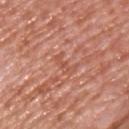No biopsy was performed on this lesion — it was imaged during a full skin examination and was not determined to be concerning. The subject is a male in their mid- to late 70s. The recorded lesion diameter is about 3 mm. Cropped from a whole-body photographic skin survey; the tile spans about 15 mm. Automated tile analysis of the lesion measured a footprint of about 3.5 mm² and an eccentricity of roughly 0.85. The software also gave roughly 6 lightness units darker than nearby skin and a normalized border contrast of about 5. The software also gave a classifier nevus-likeness of about 0/100. Captured under white-light illumination. On the upper back.